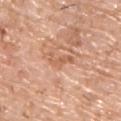workup — no biopsy performed (imaged during a skin exam)
site — the upper back
tile lighting — white-light
size — about 4 mm
subject — male, aged around 75
image source — ~15 mm tile from a whole-body skin photo